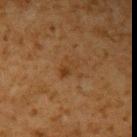  biopsy_status: not biopsied; imaged during a skin examination
  lighting: cross-polarized
  site: left upper arm
  patient:
    sex: male
    age_approx: 60
  image:
    source: total-body photography crop
    field_of_view_mm: 15
  lesion_size:
    long_diameter_mm_approx: 2.5